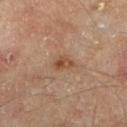The lesion's longest dimension is about 2.5 mm.
Located on the left lower leg.
A 15 mm crop from a total-body photograph taken for skin-cancer surveillance.
Automated image analysis of the tile measured a footprint of about 3.5 mm², an outline eccentricity of about 0.8 (0 = round, 1 = elongated), and two-axis asymmetry of about 0.35. And it measured a lesion–skin lightness drop of about 9 and a normalized lesion–skin contrast near 7.5.
The patient is aged 53–57.